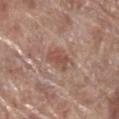Captured during whole-body skin photography for melanoma surveillance; the lesion was not biopsied. On the leg. Approximately 3.5 mm at its widest. Cropped from a total-body skin-imaging series; the visible field is about 15 mm. The lesion-visualizer software estimated a mean CIELAB color near L≈51 a*≈21 b*≈27, about 9 CIELAB-L* units darker than the surrounding skin, and a lesion-to-skin contrast of about 6.5 (normalized; higher = more distinct). The patient is a male aged approximately 70. Captured under white-light illumination.